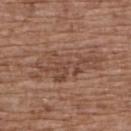illumination = white-light
site = the upper back
image = ~15 mm tile from a whole-body skin photo
patient = female, in their mid-70s
automated metrics = an area of roughly 20 mm², a shape eccentricity near 0.95, and a symmetry-axis asymmetry near 0.35